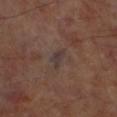{"biopsy_status": "not biopsied; imaged during a skin examination", "lesion_size": {"long_diameter_mm_approx": 3.0}, "image": {"source": "total-body photography crop", "field_of_view_mm": 15}, "automated_metrics": {"vs_skin_darker_L": 6.0, "vs_skin_contrast_norm": 7.5, "border_irregularity_0_10": 4.0, "peripheral_color_asymmetry": 0.5, "lesion_detection_confidence_0_100": 80}, "lighting": "cross-polarized", "site": "left lower leg"}